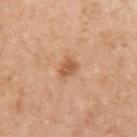Q: Illumination type?
A: white-light
Q: Lesion size?
A: ~2.5 mm (longest diameter)
Q: What did automated image analysis measure?
A: a lesion color around L≈58 a*≈24 b*≈36 in CIELAB, about 10 CIELAB-L* units darker than the surrounding skin, and a normalized border contrast of about 7; a border-irregularity rating of about 3/10, a within-lesion color-variation index near 2.5/10, and peripheral color asymmetry of about 1; a detector confidence of about 100 out of 100 that the crop contains a lesion
Q: What kind of image is this?
A: total-body-photography crop, ~15 mm field of view
Q: What is the anatomic site?
A: the left upper arm
Q: Who is the patient?
A: female, about 40 years old